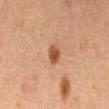The lesion was photographed on a routine skin check and not biopsied; there is no pathology result.
A roughly 15 mm field-of-view crop from a total-body skin photograph.
A female patient.
About 2.5 mm across.
On the abdomen.